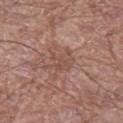biopsy status: total-body-photography surveillance lesion; no biopsy
patient: male, aged approximately 70
image: total-body-photography crop, ~15 mm field of view
location: the leg